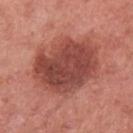Recorded during total-body skin imaging; not selected for excision or biopsy. An algorithmic analysis of the crop reported internal color variation of about 5 on a 0–10 scale and peripheral color asymmetry of about 1.5. It also reported an automated nevus-likeness rating near 80 out of 100 and a detector confidence of about 100 out of 100 that the crop contains a lesion. A 15 mm close-up extracted from a 3D total-body photography capture. The lesion is located on the left upper arm. A female subject, aged approximately 50. The lesion's longest dimension is about 8 mm. This is a white-light tile.